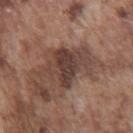• notes · no biopsy performed (imaged during a skin exam)
• patient · male, approximately 75 years of age
• tile lighting · white-light illumination
• location · the chest
• automated metrics · about 11 CIELAB-L* units darker than the surrounding skin and a lesion-to-skin contrast of about 9 (normalized; higher = more distinct); a border-irregularity rating of about 5/10 and a color-variation rating of about 5/10; a classifier nevus-likeness of about 15/100 and a detector confidence of about 95 out of 100 that the crop contains a lesion
• size · ≈6.5 mm
• image source · ~15 mm crop, total-body skin-cancer survey